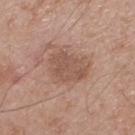workup — catalogued during a skin exam; not biopsied
anatomic site — the upper back
patient — male, in their 70s
acquisition — total-body-photography crop, ~15 mm field of view
lighting — white-light illumination
TBP lesion metrics — a mean CIELAB color near L≈53 a*≈19 b*≈27, a lesion–skin lightness drop of about 8, and a lesion-to-skin contrast of about 6 (normalized; higher = more distinct); an automated nevus-likeness rating near 0 out of 100
size — about 5 mm